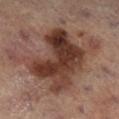| field | value |
|---|---|
| follow-up | imaged on a skin check; not biopsied |
| patient | female, in their 80s |
| imaging modality | ~15 mm crop, total-body skin-cancer survey |
| site | the left leg |